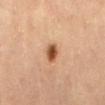biopsy status: catalogued during a skin exam; not biopsied | acquisition: ~15 mm crop, total-body skin-cancer survey | image-analysis metrics: an area of roughly 4.5 mm² and a shape eccentricity near 0.75; internal color variation of about 4 on a 0–10 scale and radial color variation of about 1 | patient: female, roughly 60 years of age | body site: the lower back | lighting: cross-polarized.